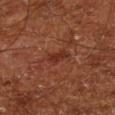follow-up = total-body-photography surveillance lesion; no biopsy | diameter = ~2.5 mm (longest diameter) | tile lighting = cross-polarized | body site = the left lower leg | subject = male, about 65 years old | image-analysis metrics = a border-irregularity rating of about 3/10, a color-variation rating of about 1/10, and radial color variation of about 0.5; an automated nevus-likeness rating near 0 out of 100 and lesion-presence confidence of about 100/100 | image = ~15 mm tile from a whole-body skin photo.